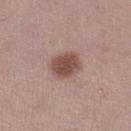biopsy status = no biopsy performed (imaged during a skin exam); patient = female, about 50 years old; lesion diameter = ≈3.5 mm; tile lighting = white-light illumination; body site = the leg; image source = ~15 mm tile from a whole-body skin photo.